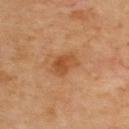No biopsy was performed on this lesion — it was imaged during a full skin examination and was not determined to be concerning. A 15 mm close-up extracted from a 3D total-body photography capture. Located on the back.